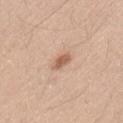{
  "biopsy_status": "not biopsied; imaged during a skin examination",
  "image": {
    "source": "total-body photography crop",
    "field_of_view_mm": 15
  },
  "patient": {
    "sex": "male",
    "age_approx": 35
  },
  "lighting": "white-light",
  "site": "left thigh",
  "lesion_size": {
    "long_diameter_mm_approx": 2.5
  }
}